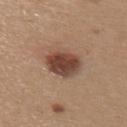Assessment: No biopsy was performed on this lesion — it was imaged during a full skin examination and was not determined to be concerning. Image and clinical context: The tile uses white-light illumination. A female subject, approximately 30 years of age. On the upper back. A roughly 15 mm field-of-view crop from a total-body skin photograph. Automated tile analysis of the lesion measured a footprint of about 12 mm² and two-axis asymmetry of about 0.15. And it measured an automated nevus-likeness rating near 95 out of 100 and a detector confidence of about 100 out of 100 that the crop contains a lesion.